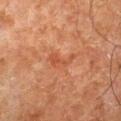No biopsy was performed on this lesion — it was imaged during a full skin examination and was not determined to be concerning.
A 15 mm crop from a total-body photograph taken for skin-cancer surveillance.
From the left thigh.
A male patient aged around 80.
Approximately 3.5 mm at its widest.